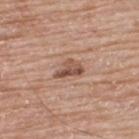Q: What lighting was used for the tile?
A: white-light
Q: What is the imaging modality?
A: total-body-photography crop, ~15 mm field of view
Q: Where on the body is the lesion?
A: the back
Q: What are the patient's age and sex?
A: male, aged 63 to 67